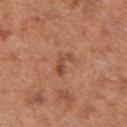Assessment: The lesion was tiled from a total-body skin photograph and was not biopsied. Context: A 15 mm close-up extracted from a 3D total-body photography capture. The patient is a male aged 63–67. The lesion's longest dimension is about 3 mm. Located on the front of the torso.